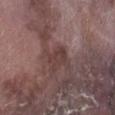Context: The tile uses white-light illumination. Longest diameter approximately 3 mm. A close-up tile cropped from a whole-body skin photograph, about 15 mm across. The lesion is on the leg. Automated tile analysis of the lesion measured an average lesion color of about L≈38 a*≈18 b*≈19 (CIELAB), about 7 CIELAB-L* units darker than the surrounding skin, and a normalized border contrast of about 6. The subject is a male in their mid- to late 70s.